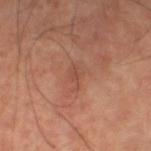Q: Is there a histopathology result?
A: catalogued during a skin exam; not biopsied
Q: How was this image acquired?
A: total-body-photography crop, ~15 mm field of view
Q: Who is the patient?
A: male, aged around 60
Q: Where on the body is the lesion?
A: the leg
Q: How was the tile lit?
A: cross-polarized illumination